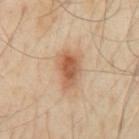notes = imaged on a skin check; not biopsied | automated lesion analysis = a nevus-likeness score of about 95/100 and a lesion-detection confidence of about 100/100 | site = the mid back | imaging modality = ~15 mm crop, total-body skin-cancer survey | subject = male, aged 53 to 57 | illumination = cross-polarized illumination.